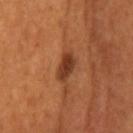Imaged during a routine full-body skin examination; the lesion was not biopsied and no histopathology is available.
Captured under cross-polarized illumination.
Approximately 4 mm at its widest.
Cropped from a whole-body photographic skin survey; the tile spans about 15 mm.
The lesion is located on the head or neck.
A male patient in their 40s.